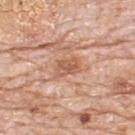Clinical summary:
On the upper back. This is a white-light tile. A region of skin cropped from a whole-body photographic capture, roughly 15 mm wide. The subject is a male aged 78–82.